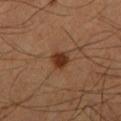The lesion was photographed on a routine skin check and not biopsied; there is no pathology result.
The subject is a male aged 53–57.
On the leg.
Automated image analysis of the tile measured a shape eccentricity near 0.5 and two-axis asymmetry of about 0.25. The analysis additionally found an average lesion color of about L≈34 a*≈21 b*≈30 (CIELAB), roughly 10 lightness units darker than nearby skin, and a normalized border contrast of about 9.5. The software also gave a border-irregularity rating of about 2/10 and internal color variation of about 2.5 on a 0–10 scale. And it measured a classifier nevus-likeness of about 95/100.
Imaged with cross-polarized lighting.
A 15 mm crop from a total-body photograph taken for skin-cancer surveillance.